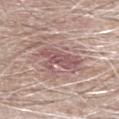Part of a total-body skin-imaging series; this lesion was reviewed on a skin check and was not flagged for biopsy. On the right forearm. A roughly 15 mm field-of-view crop from a total-body skin photograph. A male patient roughly 65 years of age.